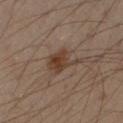Captured during whole-body skin photography for melanoma surveillance; the lesion was not biopsied.
The lesion is located on the right forearm.
A close-up tile cropped from a whole-body skin photograph, about 15 mm across.
Approximately 4.5 mm at its widest.
A male patient about 45 years old.
This is a cross-polarized tile.